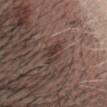Clinical impression:
No biopsy was performed on this lesion — it was imaged during a full skin examination and was not determined to be concerning.
Background:
On the head or neck. The total-body-photography lesion software estimated a color-variation rating of about 4.5/10 and a peripheral color-asymmetry measure near 2. The analysis additionally found an automated nevus-likeness rating near 55 out of 100 and a detector confidence of about 100 out of 100 that the crop contains a lesion. Cropped from a total-body skin-imaging series; the visible field is about 15 mm. Approximately 3 mm at its widest. The patient is a male roughly 40 years of age.